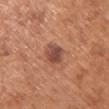notes: imaged on a skin check; not biopsied | diameter: about 3 mm | patient: female, about 65 years old | location: the front of the torso | image: total-body-photography crop, ~15 mm field of view | tile lighting: white-light.